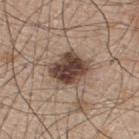workup = imaged on a skin check; not biopsied | patient = male, aged around 50 | body site = the upper back | image source = ~15 mm tile from a whole-body skin photo.